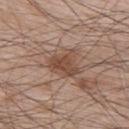Notes:
- biopsy status · total-body-photography surveillance lesion; no biopsy
- acquisition · total-body-photography crop, ~15 mm field of view
- location · the upper back
- lesion diameter · about 4 mm
- lighting · white-light
- subject · male, approximately 65 years of age